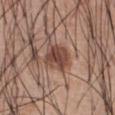Part of a total-body skin-imaging series; this lesion was reviewed on a skin check and was not flagged for biopsy. The lesion is on the abdomen. A lesion tile, about 15 mm wide, cut from a 3D total-body photograph. Automated tile analysis of the lesion measured a footprint of about 9.5 mm², an outline eccentricity of about 0.4 (0 = round, 1 = elongated), and a symmetry-axis asymmetry near 0.2. The software also gave border irregularity of about 2 on a 0–10 scale, a color-variation rating of about 4.5/10, and a peripheral color-asymmetry measure near 1.5. It also reported an automated nevus-likeness rating near 85 out of 100 and a lesion-detection confidence of about 100/100. The recorded lesion diameter is about 3.5 mm. The patient is a male aged 53 to 57. Captured under white-light illumination.